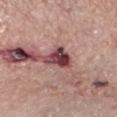<case>
<biopsy_status>not biopsied; imaged during a skin examination</biopsy_status>
<lighting>white-light</lighting>
<lesion_size>
  <long_diameter_mm_approx>3.5</long_diameter_mm_approx>
</lesion_size>
<image>
  <source>total-body photography crop</source>
  <field_of_view_mm>15</field_of_view_mm>
</image>
<patient>
  <sex>male</sex>
  <age_approx>50</age_approx>
</patient>
<site>left lower leg</site>
</case>